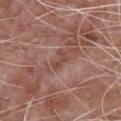No biopsy was performed on this lesion — it was imaged during a full skin examination and was not determined to be concerning. The total-body-photography lesion software estimated an area of roughly 3 mm², an outline eccentricity of about 0.85 (0 = round, 1 = elongated), and two-axis asymmetry of about 0.4. It also reported a lesion color around L≈46 a*≈21 b*≈25 in CIELAB and a lesion-to-skin contrast of about 6 (normalized; higher = more distinct). It also reported a border-irregularity index near 4.5/10, a within-lesion color-variation index near 0/10, and a peripheral color-asymmetry measure near 0. And it measured an automated nevus-likeness rating near 0 out of 100 and a detector confidence of about 95 out of 100 that the crop contains a lesion. A close-up tile cropped from a whole-body skin photograph, about 15 mm across. Captured under white-light illumination. The recorded lesion diameter is about 2.5 mm. A male subject, roughly 60 years of age. The lesion is located on the upper back.